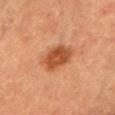The lesion was photographed on a routine skin check and not biopsied; there is no pathology result. A female subject aged 53–57. Located on the chest. Cropped from a total-body skin-imaging series; the visible field is about 15 mm.